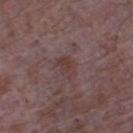Captured during whole-body skin photography for melanoma surveillance; the lesion was not biopsied.
The recorded lesion diameter is about 3 mm.
Located on the chest.
A male patient, approximately 65 years of age.
A lesion tile, about 15 mm wide, cut from a 3D total-body photograph.